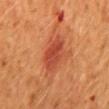A female subject aged approximately 50. About 4.5 mm across. A 15 mm close-up extracted from a 3D total-body photography capture. The lesion-visualizer software estimated an average lesion color of about L≈40 a*≈28 b*≈32 (CIELAB), roughly 8 lightness units darker than nearby skin, and a normalized lesion–skin contrast near 7. The software also gave a border-irregularity index near 2.5/10 and a color-variation rating of about 4/10. Located on the mid back.